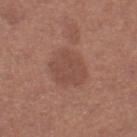image source: total-body-photography crop, ~15 mm field of view
lighting: white-light
patient: female, aged around 50
size: about 5 mm
image-analysis metrics: an area of roughly 15 mm² and an outline eccentricity of about 0.6 (0 = round, 1 = elongated); a nevus-likeness score of about 5/100
anatomic site: the right lower leg